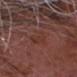follow-up — imaged on a skin check; not biopsied | location — the head or neck | patient — male, in their mid-70s | imaging modality — ~15 mm tile from a whole-body skin photo.